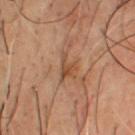This lesion was catalogued during total-body skin photography and was not selected for biopsy.
The lesion is located on the chest.
A roughly 15 mm field-of-view crop from a total-body skin photograph.
A male subject roughly 50 years of age.
This is a cross-polarized tile.